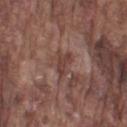Findings:
• notes — imaged on a skin check; not biopsied
• patient — male, roughly 75 years of age
• image — ~15 mm tile from a whole-body skin photo
• automated metrics — an area of roughly 4 mm², a shape eccentricity near 0.8, and a shape-asymmetry score of about 0.35 (0 = symmetric); a nevus-likeness score of about 0/100 and lesion-presence confidence of about 95/100
• lighting — white-light illumination
• diameter — about 3 mm
• anatomic site — the left upper arm